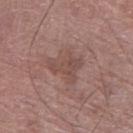Captured during whole-body skin photography for melanoma surveillance; the lesion was not biopsied. A 15 mm close-up tile from a total-body photography series done for melanoma screening. The lesion is located on the left thigh. About 4 mm across. A male patient about 75 years old. This is a white-light tile.